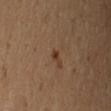Clinical impression: The lesion was tiled from a total-body skin photograph and was not biopsied. Acquisition and patient details: The lesion is on the left upper arm. Captured under cross-polarized illumination. Automated image analysis of the tile measured a border-irregularity index near 2/10 and a peripheral color-asymmetry measure near 0. A 15 mm crop from a total-body photograph taken for skin-cancer surveillance. The patient is a male roughly 45 years of age. The recorded lesion diameter is about 1 mm.